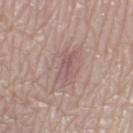follow-up: imaged on a skin check; not biopsied | image: 15 mm crop, total-body photography | diameter: ≈3.5 mm | lighting: white-light | TBP lesion metrics: border irregularity of about 3.5 on a 0–10 scale and radial color variation of about 1 | location: the right lower leg | patient: female, approximately 60 years of age.